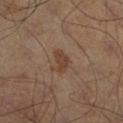Imaged during a routine full-body skin examination; the lesion was not biopsied and no histopathology is available. An algorithmic analysis of the crop reported a color-variation rating of about 1.5/10 and peripheral color asymmetry of about 0.5. The software also gave an automated nevus-likeness rating near 20 out of 100 and lesion-presence confidence of about 100/100. Imaged with cross-polarized lighting. Cropped from a total-body skin-imaging series; the visible field is about 15 mm. A male patient, aged 53–57. The lesion is on the right lower leg. About 3 mm across.